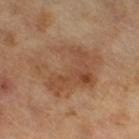| key | value |
|---|---|
| notes | no biopsy performed (imaged during a skin exam) |